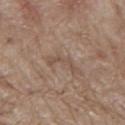| feature | finding |
|---|---|
| subject | male, aged approximately 70 |
| anatomic site | the mid back |
| automated lesion analysis | a classifier nevus-likeness of about 0/100 and lesion-presence confidence of about 95/100 |
| imaging modality | 15 mm crop, total-body photography |
| size | ~4 mm (longest diameter) |
| illumination | white-light |